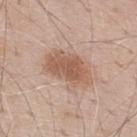The lesion was tiled from a total-body skin photograph and was not biopsied. The subject is a male aged 68–72. Longest diameter approximately 6 mm. The lesion is located on the upper back. Captured under white-light illumination. A lesion tile, about 15 mm wide, cut from a 3D total-body photograph. The total-body-photography lesion software estimated a lesion–skin lightness drop of about 10. And it measured internal color variation of about 3.5 on a 0–10 scale and radial color variation of about 1.